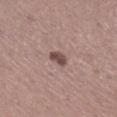Clinical impression:
This lesion was catalogued during total-body skin photography and was not selected for biopsy.